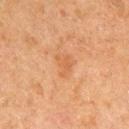workup = total-body-photography surveillance lesion; no biopsy | patient = male, aged around 65 | image source = 15 mm crop, total-body photography | location = the right upper arm.